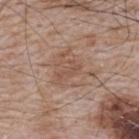The lesion was photographed on a routine skin check and not biopsied; there is no pathology result. The subject is a male about 65 years old. From the upper back. About 5 mm across. A lesion tile, about 15 mm wide, cut from a 3D total-body photograph. The total-body-photography lesion software estimated a footprint of about 14 mm² and an eccentricity of roughly 0.45. The software also gave a nevus-likeness score of about 0/100 and a detector confidence of about 95 out of 100 that the crop contains a lesion.